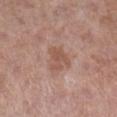| feature | finding |
|---|---|
| workup | total-body-photography surveillance lesion; no biopsy |
| patient | female, roughly 50 years of age |
| tile lighting | white-light illumination |
| imaging modality | ~15 mm crop, total-body skin-cancer survey |
| site | the right lower leg |
| size | ≈3 mm |
| automated metrics | a footprint of about 6 mm² and an eccentricity of roughly 0.5; a border-irregularity index near 5/10, a within-lesion color-variation index near 1.5/10, and radial color variation of about 0.5; an automated nevus-likeness rating near 0 out of 100 and a detector confidence of about 100 out of 100 that the crop contains a lesion |